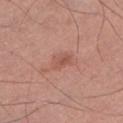* notes: catalogued during a skin exam; not biopsied
* image source: ~15 mm crop, total-body skin-cancer survey
* lesion diameter: ~3 mm (longest diameter)
* patient: male, aged approximately 60
* location: the left lower leg